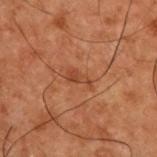Part of a total-body skin-imaging series; this lesion was reviewed on a skin check and was not flagged for biopsy. Imaged with cross-polarized lighting. From the upper back. A male patient, roughly 50 years of age. A region of skin cropped from a whole-body photographic capture, roughly 15 mm wide. The lesion-visualizer software estimated an area of roughly 3.5 mm², a shape eccentricity near 0.9, and a symmetry-axis asymmetry near 0.4. And it measured roughly 6 lightness units darker than nearby skin and a normalized lesion–skin contrast near 5.5. The software also gave an automated nevus-likeness rating near 0 out of 100 and a lesion-detection confidence of about 100/100. Longest diameter approximately 3 mm.